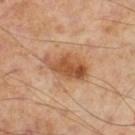The subject is a male in their mid- to late 50s. The lesion is located on the left thigh. The lesion's longest dimension is about 5 mm. Cropped from a whole-body photographic skin survey; the tile spans about 15 mm. Imaged with cross-polarized lighting.